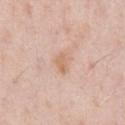lesion size — about 2.5 mm
anatomic site — the chest
patient — male, aged approximately 60
imaging modality — ~15 mm tile from a whole-body skin photo
tile lighting — white-light illumination